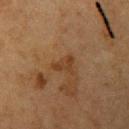The lesion was tiled from a total-body skin photograph and was not biopsied. Imaged with cross-polarized lighting. The lesion is located on the left upper arm. A roughly 15 mm field-of-view crop from a total-body skin photograph. The lesion's longest dimension is about 3 mm. Automated tile analysis of the lesion measured an automated nevus-likeness rating near 0 out of 100 and a lesion-detection confidence of about 100/100. The subject is a female in their mid- to late 50s.